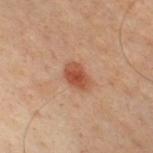{"biopsy_status": "not biopsied; imaged during a skin examination", "automated_metrics": {"border_irregularity_0_10": 2.0, "color_variation_0_10": 3.5, "peripheral_color_asymmetry": 1.0}, "site": "chest", "lighting": "cross-polarized", "patient": {"sex": "male", "age_approx": 50}, "image": {"source": "total-body photography crop", "field_of_view_mm": 15}}